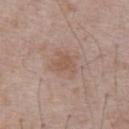Captured during whole-body skin photography for melanoma surveillance; the lesion was not biopsied. A 15 mm close-up extracted from a 3D total-body photography capture. A male subject, aged approximately 70. The lesion is on the abdomen.